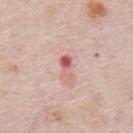| feature | finding |
|---|---|
| notes | no biopsy performed (imaged during a skin exam) |
| illumination | white-light |
| image source | ~15 mm crop, total-body skin-cancer survey |
| anatomic site | the chest |
| lesion size | about 3.5 mm |
| patient | male, roughly 80 years of age |